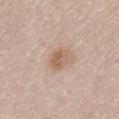Assessment:
Recorded during total-body skin imaging; not selected for excision or biopsy.
Clinical summary:
From the lower back. A region of skin cropped from a whole-body photographic capture, roughly 15 mm wide. Approximately 2.5 mm at its widest. A male patient, aged approximately 65. The lesion-visualizer software estimated a classifier nevus-likeness of about 70/100 and a detector confidence of about 100 out of 100 that the crop contains a lesion. This is a white-light tile.